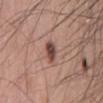Imaged during a routine full-body skin examination; the lesion was not biopsied and no histopathology is available.
Automated image analysis of the tile measured border irregularity of about 2 on a 0–10 scale, internal color variation of about 6.5 on a 0–10 scale, and peripheral color asymmetry of about 2. The analysis additionally found an automated nevus-likeness rating near 95 out of 100.
The patient is a male approximately 70 years of age.
Captured under white-light illumination.
A 15 mm close-up tile from a total-body photography series done for melanoma screening.
Measured at roughly 3 mm in maximum diameter.
On the mid back.